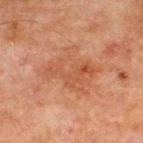subject: male, approximately 65 years of age
acquisition: ~15 mm tile from a whole-body skin photo
image-analysis metrics: a mean CIELAB color near L≈43 a*≈22 b*≈29, a lesion–skin lightness drop of about 6, and a lesion-to-skin contrast of about 5 (normalized; higher = more distinct); border irregularity of about 4.5 on a 0–10 scale, a color-variation rating of about 4/10, and radial color variation of about 1.5; a nevus-likeness score of about 0/100 and lesion-presence confidence of about 100/100
illumination: cross-polarized
location: the chest